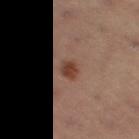The lesion was tiled from a total-body skin photograph and was not biopsied. The subject is a male in their mid-50s. On the right thigh. Automated tile analysis of the lesion measured a lesion area of about 4 mm², an outline eccentricity of about 0.7 (0 = round, 1 = elongated), and a shape-asymmetry score of about 0.2 (0 = symmetric). It also reported a border-irregularity rating of about 2/10, a within-lesion color-variation index near 2/10, and radial color variation of about 0.5. Approximately 2.5 mm at its widest. Cropped from a total-body skin-imaging series; the visible field is about 15 mm.